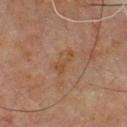biopsy status — imaged on a skin check; not biopsied | illumination — cross-polarized illumination | size — ≈3.5 mm | acquisition — 15 mm crop, total-body photography | body site — the upper back | subject — male, aged 63–67.